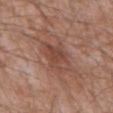Impression: Imaged during a routine full-body skin examination; the lesion was not biopsied and no histopathology is available. Acquisition and patient details: The patient is a male approximately 60 years of age. About 4.5 mm across. The lesion is located on the mid back. A 15 mm close-up tile from a total-body photography series done for melanoma screening.